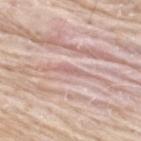Captured during whole-body skin photography for melanoma surveillance; the lesion was not biopsied.
The patient is a male about 75 years old.
Imaged with white-light lighting.
On the upper back.
The total-body-photography lesion software estimated an automated nevus-likeness rating near 0 out of 100 and a lesion-detection confidence of about 0/100.
A roughly 15 mm field-of-view crop from a total-body skin photograph.